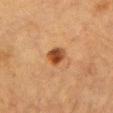Assessment:
No biopsy was performed on this lesion — it was imaged during a full skin examination and was not determined to be concerning.
Image and clinical context:
A 15 mm close-up extracted from a 3D total-body photography capture. A male patient approximately 85 years of age or older. The lesion's longest dimension is about 2.5 mm. From the chest. The lesion-visualizer software estimated border irregularity of about 2 on a 0–10 scale, a within-lesion color-variation index near 6/10, and radial color variation of about 2. The software also gave a lesion-detection confidence of about 100/100. Imaged with cross-polarized lighting.